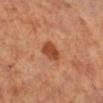A female subject aged around 40.
From the right lower leg.
A region of skin cropped from a whole-body photographic capture, roughly 15 mm wide.